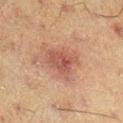Impression: No biopsy was performed on this lesion — it was imaged during a full skin examination and was not determined to be concerning. Background: Cropped from a total-body skin-imaging series; the visible field is about 15 mm. Measured at roughly 4 mm in maximum diameter. The patient is a male aged approximately 60. Imaged with cross-polarized lighting. On the right lower leg.